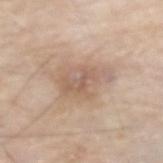The lesion was photographed on a routine skin check and not biopsied; there is no pathology result. On the left forearm. About 5.5 mm across. Captured under white-light illumination. A male subject aged around 80. This image is a 15 mm lesion crop taken from a total-body photograph.